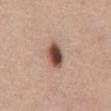Q: Is there a histopathology result?
A: no biopsy performed (imaged during a skin exam)
Q: What lighting was used for the tile?
A: white-light
Q: What is the lesion's diameter?
A: ≈4 mm
Q: What kind of image is this?
A: ~15 mm crop, total-body skin-cancer survey
Q: Where on the body is the lesion?
A: the abdomen
Q: What are the patient's age and sex?
A: male, approximately 55 years of age